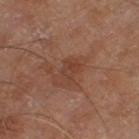Imaged during a routine full-body skin examination; the lesion was not biopsied and no histopathology is available. A male patient aged around 70. The lesion is on the left thigh. This is a cross-polarized tile. A 15 mm close-up extracted from a 3D total-body photography capture.